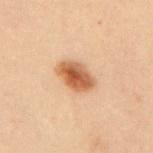Clinical impression:
The lesion was tiled from a total-body skin photograph and was not biopsied.
Acquisition and patient details:
A 15 mm close-up extracted from a 3D total-body photography capture. Captured under cross-polarized illumination. A female patient, roughly 30 years of age. Located on the mid back.